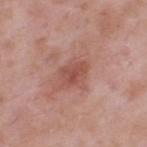  biopsy_status: not biopsied; imaged during a skin examination
  automated_metrics:
    area_mm2_approx: 5.5
    eccentricity: 0.7
    shape_asymmetry: 0.25
    vs_skin_darker_L: 9.0
    vs_skin_contrast_norm: 6.5
  patient:
    sex: male
    age_approx: 55
  site: upper back
  image:
    source: total-body photography crop
    field_of_view_mm: 15
  lesion_size:
    long_diameter_mm_approx: 3.0